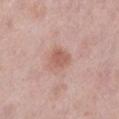- biopsy status — catalogued during a skin exam; not biopsied
- lesion diameter — about 3 mm
- automated lesion analysis — a lesion color around L≈59 a*≈23 b*≈26 in CIELAB, a lesion–skin lightness drop of about 9, and a normalized border contrast of about 6.5
- anatomic site — the left lower leg
- acquisition — total-body-photography crop, ~15 mm field of view
- subject — female, roughly 40 years of age
- lighting — white-light illumination